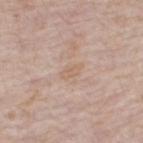biopsy_status: not biopsied; imaged during a skin examination
patient:
  sex: female
  age_approx: 60
lesion_size:
  long_diameter_mm_approx: 3.0
site: right thigh
automated_metrics:
  area_mm2_approx: 3.0
  eccentricity: 0.85
  shape_asymmetry: 0.3
  cielab_L: 62
  cielab_a: 16
  cielab_b: 29
  vs_skin_darker_L: 5.0
  vs_skin_contrast_norm: 4.5
  nevus_likeness_0_100: 0
image:
  source: total-body photography crop
  field_of_view_mm: 15